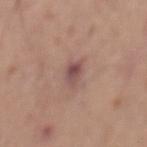workup: catalogued during a skin exam; not biopsied
patient: male, approximately 70 years of age
anatomic site: the mid back
image source: ~15 mm crop, total-body skin-cancer survey